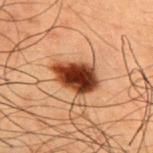Impression:
This lesion was catalogued during total-body skin photography and was not selected for biopsy.